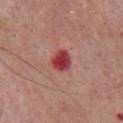Captured during whole-body skin photography for melanoma surveillance; the lesion was not biopsied.
A close-up tile cropped from a whole-body skin photograph, about 15 mm across.
The lesion is located on the front of the torso.
A male patient roughly 65 years of age.